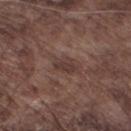biopsy status: no biopsy performed (imaged during a skin exam) | diameter: ≈2.5 mm | imaging modality: ~15 mm crop, total-body skin-cancer survey | patient: male, in their mid-70s | automated metrics: a lesion area of about 4 mm², an outline eccentricity of about 0.75 (0 = round, 1 = elongated), and two-axis asymmetry of about 0.25; a lesion–skin lightness drop of about 7 and a normalized lesion–skin contrast near 7; border irregularity of about 2.5 on a 0–10 scale and a within-lesion color-variation index near 1/10; a nevus-likeness score of about 0/100 and a detector confidence of about 95 out of 100 that the crop contains a lesion | body site: the left lower leg | tile lighting: white-light.